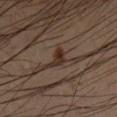{"biopsy_status": "not biopsied; imaged during a skin examination", "site": "left forearm", "image": {"source": "total-body photography crop", "field_of_view_mm": 15}, "automated_metrics": {"vs_skin_darker_L": 9.0, "vs_skin_contrast_norm": 10.0, "border_irregularity_0_10": 5.0, "color_variation_0_10": 3.0, "peripheral_color_asymmetry": 1.0}, "patient": {"sex": "male", "age_approx": 45}, "lighting": "cross-polarized"}